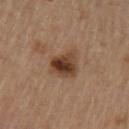{"biopsy_status": "not biopsied; imaged during a skin examination", "automated_metrics": {"eccentricity": 0.7, "shape_asymmetry": 0.25, "border_irregularity_0_10": 3.0, "color_variation_0_10": 7.5, "lesion_detection_confidence_0_100": 100}, "lesion_size": {"long_diameter_mm_approx": 4.5}, "patient": {"sex": "female", "age_approx": 45}, "site": "left upper arm", "lighting": "cross-polarized", "image": {"source": "total-body photography crop", "field_of_view_mm": 15}}